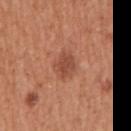Part of a total-body skin-imaging series; this lesion was reviewed on a skin check and was not flagged for biopsy.
Captured under white-light illumination.
The lesion's longest dimension is about 3 mm.
On the arm.
A male patient, in their mid- to late 50s.
Cropped from a total-body skin-imaging series; the visible field is about 15 mm.
An algorithmic analysis of the crop reported a mean CIELAB color near L≈48 a*≈26 b*≈32 and roughly 9 lightness units darker than nearby skin.